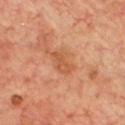Q: Was this lesion biopsied?
A: no biopsy performed (imaged during a skin exam)
Q: Where on the body is the lesion?
A: the chest
Q: How was this image acquired?
A: ~15 mm crop, total-body skin-cancer survey
Q: What did automated image analysis measure?
A: an average lesion color of about L≈53 a*≈25 b*≈36 (CIELAB), roughly 7 lightness units darker than nearby skin, and a lesion-to-skin contrast of about 5.5 (normalized; higher = more distinct); a nevus-likeness score of about 10/100 and lesion-presence confidence of about 100/100
Q: What lighting was used for the tile?
A: cross-polarized
Q: What are the patient's age and sex?
A: male, aged around 70